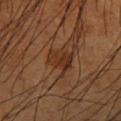Impression: Recorded during total-body skin imaging; not selected for excision or biopsy. Image and clinical context: About 4 mm across. Captured under cross-polarized illumination. A 15 mm close-up extracted from a 3D total-body photography capture. The lesion is located on the left forearm. The patient is a male in their 60s.